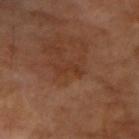This lesion was catalogued during total-body skin photography and was not selected for biopsy.
About 3 mm across.
This is a cross-polarized tile.
A 15 mm close-up extracted from a 3D total-body photography capture.
An algorithmic analysis of the crop reported an area of roughly 3 mm² and two-axis asymmetry of about 0.6.
A male patient aged around 70.
Located on the right upper arm.